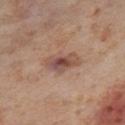notes: imaged on a skin check; not biopsied
patient: female, aged 53–57
location: the right thigh
image source: ~15 mm tile from a whole-body skin photo